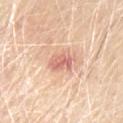Imaged during a routine full-body skin examination; the lesion was not biopsied and no histopathology is available. The subject is a male aged 63–67. From the front of the torso. Captured under white-light illumination. A lesion tile, about 15 mm wide, cut from a 3D total-body photograph. The lesion-visualizer software estimated a nevus-likeness score of about 10/100 and a detector confidence of about 100 out of 100 that the crop contains a lesion.